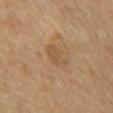biopsy_status: not biopsied; imaged during a skin examination
image:
  source: total-body photography crop
  field_of_view_mm: 15
site: chest
lesion_size:
  long_diameter_mm_approx: 3.0
patient:
  sex: male
  age_approx: 65
lighting: cross-polarized